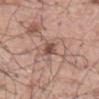{"automated_metrics": {"area_mm2_approx": 4.0, "eccentricity": 0.85, "shape_asymmetry": 0.25, "border_irregularity_0_10": 2.5, "color_variation_0_10": 4.5, "peripheral_color_asymmetry": 1.5, "nevus_likeness_0_100": 75, "lesion_detection_confidence_0_100": 100}, "site": "mid back", "lesion_size": {"long_diameter_mm_approx": 3.0}, "patient": {"sex": "male", "age_approx": 55}, "image": {"source": "total-body photography crop", "field_of_view_mm": 15}, "lighting": "white-light"}